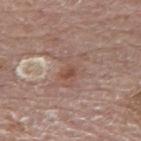Captured during whole-body skin photography for melanoma surveillance; the lesion was not biopsied.
Cropped from a total-body skin-imaging series; the visible field is about 15 mm.
A male subject, aged 78–82.
On the mid back.